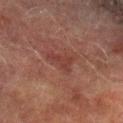{
  "biopsy_status": "not biopsied; imaged during a skin examination",
  "image": {
    "source": "total-body photography crop",
    "field_of_view_mm": 15
  },
  "lighting": "cross-polarized",
  "site": "right lower leg",
  "patient": {
    "sex": "male",
    "age_approx": 75
  },
  "lesion_size": {
    "long_diameter_mm_approx": 3.5
  },
  "automated_metrics": {
    "area_mm2_approx": 4.0,
    "eccentricity": 0.8,
    "shape_asymmetry": 0.6,
    "cielab_L": 32,
    "cielab_a": 21,
    "cielab_b": 21,
    "vs_skin_darker_L": 6.0,
    "vs_skin_contrast_norm": 5.5,
    "border_irregularity_0_10": 6.0,
    "color_variation_0_10": 1.5,
    "nevus_likeness_0_100": 0,
    "lesion_detection_confidence_0_100": 100
  }
}